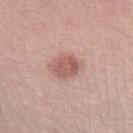Assessment: Recorded during total-body skin imaging; not selected for excision or biopsy. Image and clinical context: The lesion is located on the right lower leg. Cropped from a total-body skin-imaging series; the visible field is about 15 mm. The subject is a female aged around 20. The tile uses white-light illumination. An algorithmic analysis of the crop reported two-axis asymmetry of about 0.15. And it measured border irregularity of about 1.5 on a 0–10 scale and radial color variation of about 1. The software also gave lesion-presence confidence of about 100/100. Approximately 3.5 mm at its widest.